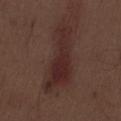Part of a total-body skin-imaging series; this lesion was reviewed on a skin check and was not flagged for biopsy. The lesion is on the abdomen. The lesion-visualizer software estimated an area of roughly 20 mm², an eccentricity of roughly 0.95, and two-axis asymmetry of about 0.45. It also reported a within-lesion color-variation index near 4/10 and peripheral color asymmetry of about 1. The analysis additionally found a nevus-likeness score of about 20/100 and a lesion-detection confidence of about 100/100. A male patient, approximately 70 years of age. Imaged with white-light lighting. A close-up tile cropped from a whole-body skin photograph, about 15 mm across.